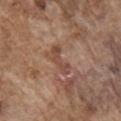The lesion was photographed on a routine skin check and not biopsied; there is no pathology result. The total-body-photography lesion software estimated a border-irregularity rating of about 6.5/10 and internal color variation of about 3 on a 0–10 scale. The software also gave a classifier nevus-likeness of about 0/100. The patient is a male aged 73 to 77. This is a white-light tile. About 4 mm across. A roughly 15 mm field-of-view crop from a total-body skin photograph. On the right upper arm.